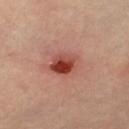lesion diameter: ≈3 mm
patient: female, approximately 65 years of age
image: ~15 mm crop, total-body skin-cancer survey
automated lesion analysis: a lesion color around L≈46 a*≈31 b*≈30 in CIELAB, about 14 CIELAB-L* units darker than the surrounding skin, and a lesion-to-skin contrast of about 10.5 (normalized; higher = more distinct); a border-irregularity rating of about 1.5/10, internal color variation of about 10 on a 0–10 scale, and radial color variation of about 3.5
illumination: cross-polarized illumination
site: the left thigh